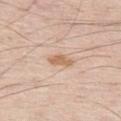Q: Is there a histopathology result?
A: catalogued during a skin exam; not biopsied
Q: Who is the patient?
A: male, approximately 60 years of age
Q: How was the tile lit?
A: white-light
Q: What did automated image analysis measure?
A: two-axis asymmetry of about 0.3; border irregularity of about 3 on a 0–10 scale, internal color variation of about 2 on a 0–10 scale, and radial color variation of about 0.5; an automated nevus-likeness rating near 50 out of 100 and a detector confidence of about 100 out of 100 that the crop contains a lesion
Q: What kind of image is this?
A: 15 mm crop, total-body photography
Q: What is the lesion's diameter?
A: ≈3.5 mm
Q: What is the anatomic site?
A: the left thigh